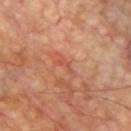biopsy status=catalogued during a skin exam; not biopsied | body site=the chest | acquisition=~15 mm crop, total-body skin-cancer survey | lesion size=≈3 mm | tile lighting=cross-polarized | subject=male, aged approximately 60.